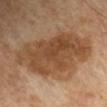The lesion was photographed on a routine skin check and not biopsied; there is no pathology result. A male patient about 60 years old. A close-up tile cropped from a whole-body skin photograph, about 15 mm across. From the right upper arm. Captured under cross-polarized illumination.